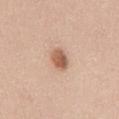The lesion was photographed on a routine skin check and not biopsied; there is no pathology result. Located on the abdomen. Cropped from a total-body skin-imaging series; the visible field is about 15 mm. About 3 mm across. Captured under white-light illumination. The subject is a male aged around 35.